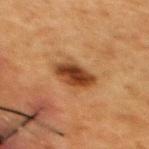Clinical impression: Imaged during a routine full-body skin examination; the lesion was not biopsied and no histopathology is available. Background: Located on the upper back. The subject is a female in their 60s. A region of skin cropped from a whole-body photographic capture, roughly 15 mm wide.